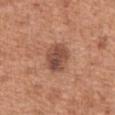Q: Was this lesion biopsied?
A: no biopsy performed (imaged during a skin exam)
Q: Who is the patient?
A: male, approximately 65 years of age
Q: What lighting was used for the tile?
A: white-light
Q: What is the imaging modality?
A: total-body-photography crop, ~15 mm field of view
Q: Where on the body is the lesion?
A: the abdomen